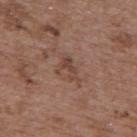The lesion was photographed on a routine skin check and not biopsied; there is no pathology result.
The lesion's longest dimension is about 3.5 mm.
Located on the upper back.
A 15 mm close-up tile from a total-body photography series done for melanoma screening.
A female subject, aged 63–67.
This is a white-light tile.
Automated tile analysis of the lesion measured a lesion area of about 4 mm², an eccentricity of roughly 0.85, and a shape-asymmetry score of about 0.55 (0 = symmetric). The software also gave an automated nevus-likeness rating near 0 out of 100 and a lesion-detection confidence of about 100/100.